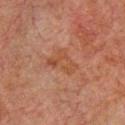No biopsy was performed on this lesion — it was imaged during a full skin examination and was not determined to be concerning. An algorithmic analysis of the crop reported an eccentricity of roughly 0.7 and a shape-asymmetry score of about 0.5 (0 = symmetric). And it measured an average lesion color of about L≈37 a*≈19 b*≈27 (CIELAB), about 5 CIELAB-L* units darker than the surrounding skin, and a normalized border contrast of about 5.5. A region of skin cropped from a whole-body photographic capture, roughly 15 mm wide. Captured under cross-polarized illumination. From the chest. The lesion's longest dimension is about 4 mm. A male subject, roughly 60 years of age.